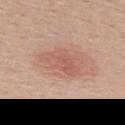{
  "biopsy_status": "not biopsied; imaged during a skin examination",
  "image": {
    "source": "total-body photography crop",
    "field_of_view_mm": 15
  },
  "patient": {
    "sex": "female",
    "age_approx": 40
  },
  "lighting": "white-light",
  "site": "back"
}